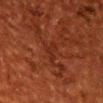Case summary:
- subject: male, approximately 60 years of age
- lighting: cross-polarized
- image source: 15 mm crop, total-body photography
- body site: the head or neck
- lesion diameter: ≈3.5 mm